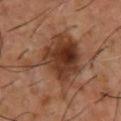workup: catalogued during a skin exam; not biopsied
lighting: cross-polarized illumination
body site: the chest
image source: ~15 mm tile from a whole-body skin photo
patient: male, aged approximately 55
automated metrics: a footprint of about 31 mm², an eccentricity of roughly 0.45, and two-axis asymmetry of about 0.45; a mean CIELAB color near L≈37 a*≈21 b*≈29, roughly 12 lightness units darker than nearby skin, and a normalized lesion–skin contrast near 9.5; a border-irregularity rating of about 6.5/10, a within-lesion color-variation index near 8/10, and radial color variation of about 3